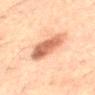workup: imaged on a skin check; not biopsied
imaging modality: ~15 mm tile from a whole-body skin photo
illumination: cross-polarized
anatomic site: the mid back
subject: male, about 50 years old